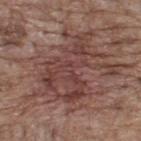No biopsy was performed on this lesion — it was imaged during a full skin examination and was not determined to be concerning. This image is a 15 mm lesion crop taken from a total-body photograph. Automated image analysis of the tile measured about 8 CIELAB-L* units darker than the surrounding skin and a normalized border contrast of about 7. The analysis additionally found a border-irregularity rating of about 8/10. The lesion is located on the upper back. The subject is a male aged 68 to 72.